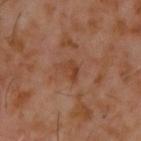Impression: Recorded during total-body skin imaging; not selected for excision or biopsy. Clinical summary: Longest diameter approximately 2.5 mm. The total-body-photography lesion software estimated a footprint of about 3.5 mm², an outline eccentricity of about 0.7 (0 = round, 1 = elongated), and a symmetry-axis asymmetry near 0.45. The software also gave a lesion color around L≈38 a*≈21 b*≈30 in CIELAB, a lesion–skin lightness drop of about 6, and a normalized lesion–skin contrast near 6.5. The software also gave a color-variation rating of about 2.5/10. A 15 mm crop from a total-body photograph taken for skin-cancer surveillance. The tile uses cross-polarized illumination. The patient is a male aged 58 to 62. The lesion is located on the upper back.